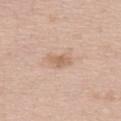| feature | finding |
|---|---|
| notes | imaged on a skin check; not biopsied |
| illumination | white-light |
| imaging modality | total-body-photography crop, ~15 mm field of view |
| patient | female, aged around 50 |
| diameter | ≈2.5 mm |
| location | the upper back |